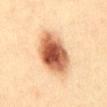Part of a total-body skin-imaging series; this lesion was reviewed on a skin check and was not flagged for biopsy. This image is a 15 mm lesion crop taken from a total-body photograph. Measured at roughly 6 mm in maximum diameter. The patient is a male roughly 40 years of age. Imaged with cross-polarized lighting. The lesion is on the abdomen.